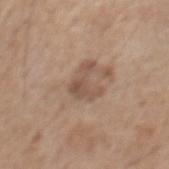Impression: No biopsy was performed on this lesion — it was imaged during a full skin examination and was not determined to be concerning. Background: An algorithmic analysis of the crop reported a footprint of about 6 mm², a shape eccentricity near 0.75, and a shape-asymmetry score of about 0.7 (0 = symmetric). The software also gave a nevus-likeness score of about 15/100 and lesion-presence confidence of about 100/100. The lesion's longest dimension is about 3.5 mm. From the front of the torso. The tile uses white-light illumination. A 15 mm close-up extracted from a 3D total-body photography capture. The patient is a male aged approximately 70.